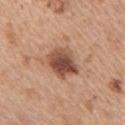The lesion is located on the chest. Captured under white-light illumination. An algorithmic analysis of the crop reported a lesion area of about 11 mm², an eccentricity of roughly 0.6, and two-axis asymmetry of about 0.25. It also reported about 15 CIELAB-L* units darker than the surrounding skin and a lesion-to-skin contrast of about 10.5 (normalized; higher = more distinct). Measured at roughly 4 mm in maximum diameter. A female patient about 45 years old. A 15 mm close-up extracted from a 3D total-body photography capture.